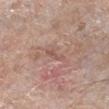workup = no biopsy performed (imaged during a skin exam) | lesion size = ~3 mm (longest diameter) | body site = the right lower leg | lighting = white-light | patient = male, aged 73 to 77 | image = ~15 mm crop, total-body skin-cancer survey.